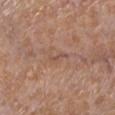<lesion>
<biopsy_status>not biopsied; imaged during a skin examination</biopsy_status>
<site>left lower leg</site>
<patient>
  <sex>female</sex>
  <age_approx>55</age_approx>
</patient>
<lighting>white-light</lighting>
<image>
  <source>total-body photography crop</source>
  <field_of_view_mm>15</field_of_view_mm>
</image>
<lesion_size>
  <long_diameter_mm_approx>3.0</long_diameter_mm_approx>
</lesion_size>
</lesion>